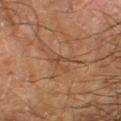Recorded during total-body skin imaging; not selected for excision or biopsy. A 15 mm close-up extracted from a 3D total-body photography capture. The subject is a male aged around 65. This is a cross-polarized tile. Measured at roughly 3 mm in maximum diameter. The lesion is located on the left lower leg.